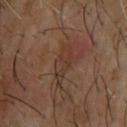workup: imaged on a skin check; not biopsied
image-analysis metrics: a border-irregularity rating of about 4.5/10, a color-variation rating of about 3/10, and radial color variation of about 1; a nevus-likeness score of about 0/100 and lesion-presence confidence of about 65/100
image: 15 mm crop, total-body photography
location: the upper back
lesion diameter: ≈5 mm
subject: male, aged approximately 65